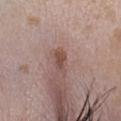Notes:
– notes — catalogued during a skin exam; not biopsied
– illumination — white-light illumination
– TBP lesion metrics — a footprint of about 3.5 mm² and a shape-asymmetry score of about 0.35 (0 = symmetric)
– site — the head or neck
– patient — female, aged 18 to 22
– acquisition — 15 mm crop, total-body photography